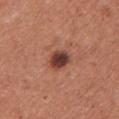The lesion is on the left upper arm.
The recorded lesion diameter is about 3 mm.
A female patient, approximately 55 years of age.
A region of skin cropped from a whole-body photographic capture, roughly 15 mm wide.
This is a white-light tile.
Automated image analysis of the tile measured an area of roughly 5.5 mm², a shape eccentricity near 0.45, and a shape-asymmetry score of about 0.15 (0 = symmetric). The analysis additionally found a mean CIELAB color near L≈40 a*≈24 b*≈26 and roughly 16 lightness units darker than nearby skin. It also reported a border-irregularity index near 1.5/10 and a within-lesion color-variation index near 5.5/10. It also reported a classifier nevus-likeness of about 75/100 and a lesion-detection confidence of about 100/100.